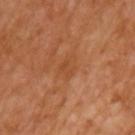Impression: This lesion was catalogued during total-body skin photography and was not selected for biopsy. Image and clinical context: Cropped from a total-body skin-imaging series; the visible field is about 15 mm. Imaged with cross-polarized lighting. About 3 mm across. From the left upper arm. A female subject, approximately 55 years of age.